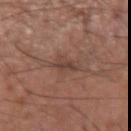| key | value |
|---|---|
| tile lighting | white-light illumination |
| location | the right forearm |
| image | total-body-photography crop, ~15 mm field of view |
| subject | male, about 55 years old |